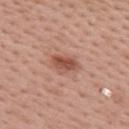Clinical impression: Captured during whole-body skin photography for melanoma surveillance; the lesion was not biopsied. Clinical summary: Cropped from a whole-body photographic skin survey; the tile spans about 15 mm. The tile uses white-light illumination. Automated image analysis of the tile measured a lesion area of about 6 mm², a shape eccentricity near 0.75, and two-axis asymmetry of about 0.35. The analysis additionally found a border-irregularity index near 3/10 and a within-lesion color-variation index near 4/10. And it measured a classifier nevus-likeness of about 90/100. Approximately 3.5 mm at its widest. The subject is a female aged 33–37. Located on the upper back.